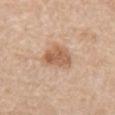– notes — total-body-photography surveillance lesion; no biopsy
– automated metrics — a lesion-to-skin contrast of about 7.5 (normalized; higher = more distinct); a border-irregularity index near 2.5/10 and a within-lesion color-variation index near 3.5/10
– patient — male, about 70 years old
– image source — 15 mm crop, total-body photography
– tile lighting — white-light
– lesion diameter — ~4 mm (longest diameter)
– anatomic site — the chest